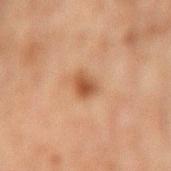Captured during whole-body skin photography for melanoma surveillance; the lesion was not biopsied. A 15 mm close-up extracted from a 3D total-body photography capture. The lesion is located on the right forearm. The subject is a male in their mid- to late 40s.